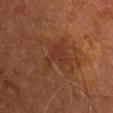{"biopsy_status": "not biopsied; imaged during a skin examination", "image": {"source": "total-body photography crop", "field_of_view_mm": 15}, "site": "right upper arm", "automated_metrics": {"area_mm2_approx": 9.0, "eccentricity": 0.75, "shape_asymmetry": 0.55, "vs_skin_darker_L": 4.0, "lesion_detection_confidence_0_100": 100}, "patient": {"sex": "male", "age_approx": 65}, "lighting": "cross-polarized", "lesion_size": {"long_diameter_mm_approx": 4.5}}